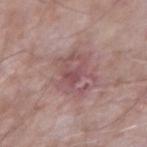On the right upper arm.
A male subject in their mid- to late 60s.
A lesion tile, about 15 mm wide, cut from a 3D total-body photograph.